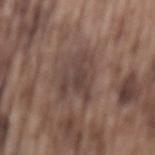Case summary:
– biopsy status — no biopsy performed (imaged during a skin exam)
– lighting — white-light
– acquisition — total-body-photography crop, ~15 mm field of view
– body site — the mid back
– subject — male, aged around 75